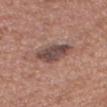workup: imaged on a skin check; not biopsied
subject: male, in their mid-40s
anatomic site: the head or neck
image source: 15 mm crop, total-body photography